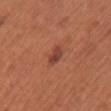| field | value |
|---|---|
| follow-up | catalogued during a skin exam; not biopsied |
| lesion diameter | ~3 mm (longest diameter) |
| imaging modality | total-body-photography crop, ~15 mm field of view |
| subject | female, aged 48–52 |
| body site | the chest |